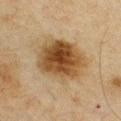Findings:
– follow-up — total-body-photography surveillance lesion; no biopsy
– illumination — cross-polarized illumination
– acquisition — ~15 mm crop, total-body skin-cancer survey
– anatomic site — the chest
– patient — male, in their mid- to late 60s
– lesion size — about 6.5 mm